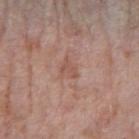Assessment: The lesion was tiled from a total-body skin photograph and was not biopsied. Context: A region of skin cropped from a whole-body photographic capture, roughly 15 mm wide. The subject is a female aged 68–72. The lesion is located on the right forearm.